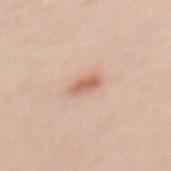biopsy status=catalogued during a skin exam; not biopsied | tile lighting=white-light | body site=the mid back | acquisition=~15 mm tile from a whole-body skin photo | subject=female, in their 40s | lesion diameter=about 3 mm.